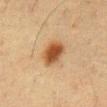Captured during whole-body skin photography for melanoma surveillance; the lesion was not biopsied. The patient is a male aged approximately 60. Located on the chest. A close-up tile cropped from a whole-body skin photograph, about 15 mm across.